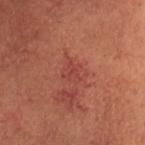Assessment: This lesion was catalogued during total-body skin photography and was not selected for biopsy. Context: From the head or neck. The lesion's longest dimension is about 2.5 mm. Captured under cross-polarized illumination. A male subject, aged 48 to 52. Automated image analysis of the tile measured an area of roughly 4 mm², a shape eccentricity near 0.5, and a symmetry-axis asymmetry near 0.35. It also reported border irregularity of about 3.5 on a 0–10 scale. This image is a 15 mm lesion crop taken from a total-body photograph.